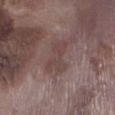Q: Was a biopsy performed?
A: total-body-photography surveillance lesion; no biopsy
Q: Where on the body is the lesion?
A: the left lower leg
Q: What did automated image analysis measure?
A: an area of roughly 9 mm² and a shape eccentricity near 0.8; a border-irregularity rating of about 4.5/10, internal color variation of about 3.5 on a 0–10 scale, and radial color variation of about 1.5; lesion-presence confidence of about 95/100
Q: Illumination type?
A: white-light illumination
Q: Lesion size?
A: ~4 mm (longest diameter)
Q: Patient demographics?
A: male, aged approximately 75
Q: How was this image acquired?
A: ~15 mm crop, total-body skin-cancer survey